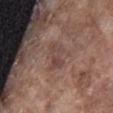Context: Imaged with white-light lighting. A 15 mm crop from a total-body photograph taken for skin-cancer surveillance. The lesion-visualizer software estimated an average lesion color of about L≈44 a*≈18 b*≈22 (CIELAB) and roughly 6 lightness units darker than nearby skin. The lesion is on the abdomen. A male patient, approximately 75 years of age.